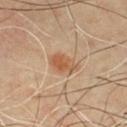| feature | finding |
|---|---|
| follow-up | no biopsy performed (imaged during a skin exam) |
| acquisition | ~15 mm crop, total-body skin-cancer survey |
| anatomic site | the chest |
| patient | male, about 55 years old |